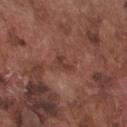{
  "biopsy_status": "not biopsied; imaged during a skin examination",
  "lesion_size": {
    "long_diameter_mm_approx": 2.5
  },
  "patient": {
    "sex": "male",
    "age_approx": 75
  },
  "image": {
    "source": "total-body photography crop",
    "field_of_view_mm": 15
  },
  "site": "chest"
}